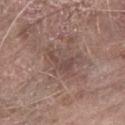Imaged during a routine full-body skin examination; the lesion was not biopsied and no histopathology is available. The lesion is located on the left forearm. Cropped from a total-body skin-imaging series; the visible field is about 15 mm. Imaged with white-light lighting. A male patient, in their 80s.